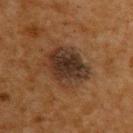{"biopsy_status": "not biopsied; imaged during a skin examination", "automated_metrics": {"area_mm2_approx": 15.0, "eccentricity": 0.6, "shape_asymmetry": 0.2, "cielab_L": 26, "cielab_a": 14, "cielab_b": 23, "vs_skin_darker_L": 10.0, "vs_skin_contrast_norm": 11.5, "lesion_detection_confidence_0_100": 100}, "image": {"source": "total-body photography crop", "field_of_view_mm": 15}, "patient": {"sex": "male", "age_approx": 60}, "site": "upper back"}